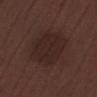Cropped from a whole-body photographic skin survey; the tile spans about 15 mm. The lesion is located on the right lower leg. Imaged with white-light lighting. About 6 mm across. A male subject, roughly 70 years of age.